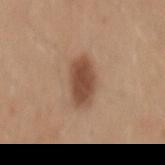<tbp_lesion>
<patient>
  <sex>male</sex>
  <age_approx>30</age_approx>
</patient>
<lesion_size>
  <long_diameter_mm_approx>4.5</long_diameter_mm_approx>
</lesion_size>
<image>
  <source>total-body photography crop</source>
  <field_of_view_mm>15</field_of_view_mm>
</image>
<lighting>white-light</lighting>
<site>back</site>
</tbp_lesion>